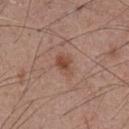location: the chest
image source: ~15 mm tile from a whole-body skin photo
size: about 2.5 mm
subject: male, in their mid- to late 50s